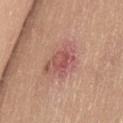Q: Was this lesion biopsied?
A: imaged on a skin check; not biopsied
Q: What lighting was used for the tile?
A: white-light
Q: What did automated image analysis measure?
A: an eccentricity of roughly 0.7 and two-axis asymmetry of about 0.3; border irregularity of about 3 on a 0–10 scale, internal color variation of about 6 on a 0–10 scale, and a peripheral color-asymmetry measure near 2; a lesion-detection confidence of about 100/100
Q: Lesion location?
A: the left thigh
Q: Lesion size?
A: ~5 mm (longest diameter)
Q: How was this image acquired?
A: ~15 mm crop, total-body skin-cancer survey
Q: Patient demographics?
A: female, about 40 years old